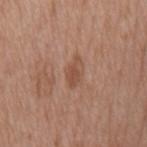Imaged during a routine full-body skin examination; the lesion was not biopsied and no histopathology is available. This is a white-light tile. A male subject about 75 years old. A 15 mm close-up tile from a total-body photography series done for melanoma screening. On the mid back.